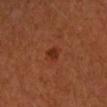notes = imaged on a skin check; not biopsied.